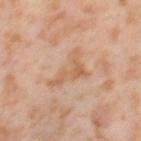The lesion was tiled from a total-body skin photograph and was not biopsied. Measured at roughly 5.5 mm in maximum diameter. Located on the left thigh. A roughly 15 mm field-of-view crop from a total-body skin photograph. A female patient aged approximately 55. This is a cross-polarized tile.